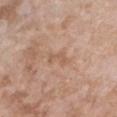Clinical impression: This lesion was catalogued during total-body skin photography and was not selected for biopsy. Context: A roughly 15 mm field-of-view crop from a total-body skin photograph. Captured under white-light illumination. The total-body-photography lesion software estimated a border-irregularity index near 6/10 and internal color variation of about 0 on a 0–10 scale. The software also gave an automated nevus-likeness rating near 0 out of 100 and a lesion-detection confidence of about 100/100. Approximately 3 mm at its widest. A female patient, aged 73–77. The lesion is located on the chest.